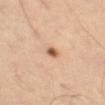biopsy status = imaged on a skin check; not biopsied
automated metrics = a mean CIELAB color near L≈55 a*≈22 b*≈33 and a lesion–skin lightness drop of about 15; border irregularity of about 1.5 on a 0–10 scale, a color-variation rating of about 2/10, and a peripheral color-asymmetry measure near 0.5; an automated nevus-likeness rating near 95 out of 100 and a lesion-detection confidence of about 100/100
size = ~1.5 mm (longest diameter)
image source = ~15 mm crop, total-body skin-cancer survey
lighting = cross-polarized
subject = male, aged 53 to 57
location = the leg